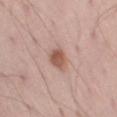Assessment:
The lesion was photographed on a routine skin check and not biopsied; there is no pathology result.
Context:
A male patient aged approximately 55. A roughly 15 mm field-of-view crop from a total-body skin photograph. Approximately 3 mm at its widest. From the left thigh.